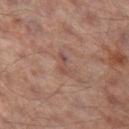Notes:
* follow-up: imaged on a skin check; not biopsied